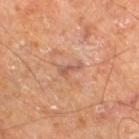Impression:
The lesion was tiled from a total-body skin photograph and was not biopsied.
Background:
A region of skin cropped from a whole-body photographic capture, roughly 15 mm wide. A male subject, in their mid-60s. Captured under cross-polarized illumination. The recorded lesion diameter is about 3 mm. The lesion is on the right thigh. The lesion-visualizer software estimated a mean CIELAB color near L≈53 a*≈22 b*≈29. It also reported border irregularity of about 5 on a 0–10 scale, a within-lesion color-variation index near 0/10, and peripheral color asymmetry of about 0.